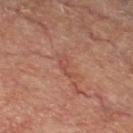biopsy status — catalogued during a skin exam; not biopsied | acquisition — 15 mm crop, total-body photography | lighting — cross-polarized | anatomic site — the left thigh | automated lesion analysis — an area of roughly 3 mm², an outline eccentricity of about 0.9 (0 = round, 1 = elongated), and a symmetry-axis asymmetry near 0.5; a lesion color around L≈48 a*≈25 b*≈28 in CIELAB and a normalized lesion–skin contrast near 4.5; a border-irregularity rating of about 6/10 and a color-variation rating of about 0.5/10 | patient — male, aged 68 to 72.